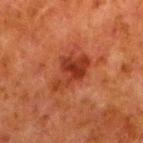{"automated_metrics": {"cielab_L": 31, "cielab_a": 27, "cielab_b": 30, "vs_skin_darker_L": 8.0, "border_irregularity_0_10": 4.5, "peripheral_color_asymmetry": 1.5}, "lighting": "cross-polarized", "lesion_size": {"long_diameter_mm_approx": 5.0}, "site": "left lower leg", "patient": {"sex": "male", "age_approx": 80}, "image": {"source": "total-body photography crop", "field_of_view_mm": 15}}